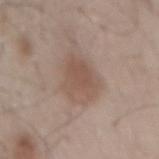workup: catalogued during a skin exam; not biopsied | imaging modality: 15 mm crop, total-body photography | automated lesion analysis: an area of roughly 14 mm², a shape eccentricity near 0.7, and two-axis asymmetry of about 0.2; a lesion color around L≈53 a*≈16 b*≈25 in CIELAB, roughly 8 lightness units darker than nearby skin, and a lesion-to-skin contrast of about 6 (normalized; higher = more distinct) | lighting: white-light illumination | body site: the mid back | size: about 5 mm | patient: male, aged 53 to 57.